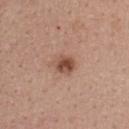{
  "biopsy_status": "not biopsied; imaged during a skin examination",
  "site": "upper back",
  "image": {
    "source": "total-body photography crop",
    "field_of_view_mm": 15
  },
  "patient": {
    "sex": "female",
    "age_approx": 40
  }
}